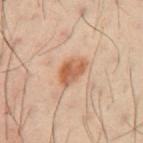No biopsy was performed on this lesion — it was imaged during a full skin examination and was not determined to be concerning.
This image is a 15 mm lesion crop taken from a total-body photograph.
The lesion is on the left upper arm.
The patient is a male in their 40s.